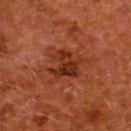No biopsy was performed on this lesion — it was imaged during a full skin examination and was not determined to be concerning. Imaged with cross-polarized lighting. From the upper back. A 15 mm close-up tile from a total-body photography series done for melanoma screening. A female subject aged approximately 50.